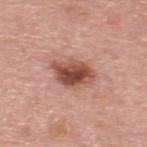The lesion was photographed on a routine skin check and not biopsied; there is no pathology result.
A male subject in their mid-60s.
The tile uses white-light illumination.
Longest diameter approximately 5 mm.
A 15 mm close-up extracted from a 3D total-body photography capture.
On the upper back.